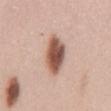Recorded during total-body skin imaging; not selected for excision or biopsy. Imaged with white-light lighting. A 15 mm close-up tile from a total-body photography series done for melanoma screening. The lesion is located on the abdomen. A male subject aged approximately 45. The lesion-visualizer software estimated a lesion area of about 11 mm², a shape eccentricity near 0.85, and two-axis asymmetry of about 0.25. The software also gave a lesion color around L≈54 a*≈21 b*≈27 in CIELAB, a lesion–skin lightness drop of about 19, and a normalized lesion–skin contrast near 12. It also reported a border-irregularity rating of about 2.5/10, internal color variation of about 6 on a 0–10 scale, and radial color variation of about 1.5. Approximately 5 mm at its widest.